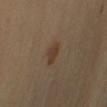<case>
<biopsy_status>not biopsied; imaged during a skin examination</biopsy_status>
</case>